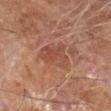| feature | finding |
|---|---|
| illumination | cross-polarized illumination |
| patient | male, in their 60s |
| size | ~4.5 mm (longest diameter) |
| site | the left forearm |
| acquisition | 15 mm crop, total-body photography |
| automated metrics | a nevus-likeness score of about 0/100 and lesion-presence confidence of about 100/100 |